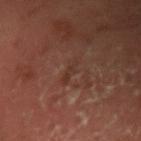- biopsy status — imaged on a skin check; not biopsied
- imaging modality — 15 mm crop, total-body photography
- patient — male, about 60 years old
- body site — the left upper arm